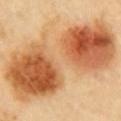Impression: Imaged during a routine full-body skin examination; the lesion was not biopsied and no histopathology is available. Background: Captured under cross-polarized illumination. The subject is a female in their 60s. The lesion is on the front of the torso. A roughly 15 mm field-of-view crop from a total-body skin photograph. Longest diameter approximately 14.5 mm.